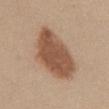Clinical impression:
No biopsy was performed on this lesion — it was imaged during a full skin examination and was not determined to be concerning.
Acquisition and patient details:
The total-body-photography lesion software estimated two-axis asymmetry of about 0.2. The software also gave a border-irregularity index near 2/10, a within-lesion color-variation index near 3.5/10, and peripheral color asymmetry of about 1. A female subject about 40 years old. A close-up tile cropped from a whole-body skin photograph, about 15 mm across. This is a white-light tile. From the abdomen.